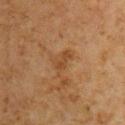Imaged during a routine full-body skin examination; the lesion was not biopsied and no histopathology is available.